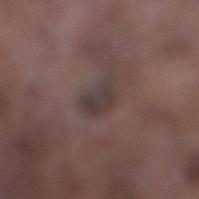{"biopsy_status": "not biopsied; imaged during a skin examination", "automated_metrics": {"area_mm2_approx": 5.5, "eccentricity": 0.8, "shape_asymmetry": 0.35, "cielab_L": 36, "cielab_a": 13, "cielab_b": 15, "vs_skin_darker_L": 7.0, "color_variation_0_10": 2.0, "peripheral_color_asymmetry": 0.5, "nevus_likeness_0_100": 0}, "image": {"source": "total-body photography crop", "field_of_view_mm": 15}, "lighting": "white-light", "patient": {"sex": "male", "age_approx": 75}, "lesion_size": {"long_diameter_mm_approx": 3.5}, "site": "right lower leg"}